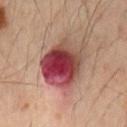Recorded during total-body skin imaging; not selected for excision or biopsy.
A close-up tile cropped from a whole-body skin photograph, about 15 mm across.
The lesion is on the mid back.
A male patient aged around 50.
The lesion's longest dimension is about 6 mm.
The tile uses cross-polarized illumination.